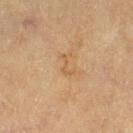{
  "biopsy_status": "not biopsied; imaged during a skin examination",
  "patient": {
    "sex": "female",
    "age_approx": 80
  },
  "image": {
    "source": "total-body photography crop",
    "field_of_view_mm": 15
  },
  "site": "right thigh",
  "lesion_size": {
    "long_diameter_mm_approx": 2.5
  },
  "automated_metrics": {
    "eccentricity": 0.9,
    "shape_asymmetry": 0.65,
    "border_irregularity_0_10": 7.5,
    "color_variation_0_10": 0.0,
    "peripheral_color_asymmetry": 0.0
  }
}